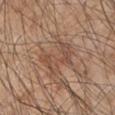This lesion was catalogued during total-body skin photography and was not selected for biopsy. The lesion's longest dimension is about 4.5 mm. A male subject, about 45 years old. A roughly 15 mm field-of-view crop from a total-body skin photograph. The tile uses white-light illumination. The lesion is on the right upper arm.